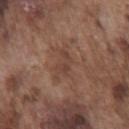Impression:
Imaged during a routine full-body skin examination; the lesion was not biopsied and no histopathology is available.
Clinical summary:
A lesion tile, about 15 mm wide, cut from a 3D total-body photograph. Approximately 2.5 mm at its widest. The subject is a male in their mid- to late 70s. Imaged with white-light lighting. Located on the chest.